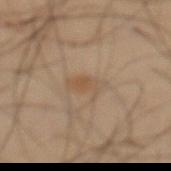workup: no biopsy performed (imaged during a skin exam); lesion size: ≈3.5 mm; subject: male, approximately 55 years of age; body site: the abdomen; image source: 15 mm crop, total-body photography; illumination: cross-polarized illumination; automated lesion analysis: lesion-presence confidence of about 100/100.